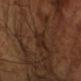Q: Was a biopsy performed?
A: total-body-photography surveillance lesion; no biopsy
Q: What did automated image analysis measure?
A: an average lesion color of about L≈24 a*≈17 b*≈25 (CIELAB), roughly 5 lightness units darker than nearby skin, and a normalized lesion–skin contrast near 6
Q: What is the imaging modality?
A: total-body-photography crop, ~15 mm field of view
Q: What is the lesion's diameter?
A: ≈2.5 mm
Q: What lighting was used for the tile?
A: cross-polarized
Q: Who is the patient?
A: male, aged 63 to 67